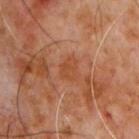The lesion was tiled from a total-body skin photograph and was not biopsied.
Cropped from a total-body skin-imaging series; the visible field is about 15 mm.
The subject is a male approximately 60 years of age.
Longest diameter approximately 3 mm.
From the chest.